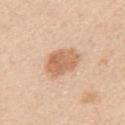automated_metrics:
  area_mm2_approx: 11.0
  border_irregularity_0_10: 1.5
  color_variation_0_10: 3.5
  peripheral_color_asymmetry: 1.0
  nevus_likeness_0_100: 30
  lesion_detection_confidence_0_100: 100
patient:
  sex: male
  age_approx: 65
site: left upper arm
lesion_size:
  long_diameter_mm_approx: 4.5
image:
  source: total-body photography crop
  field_of_view_mm: 15